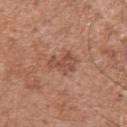Q: Was a biopsy performed?
A: no biopsy performed (imaged during a skin exam)
Q: What kind of image is this?
A: ~15 mm tile from a whole-body skin photo
Q: Patient demographics?
A: male, approximately 75 years of age
Q: How was the tile lit?
A: white-light
Q: Lesion size?
A: about 3.5 mm
Q: Where on the body is the lesion?
A: the right upper arm